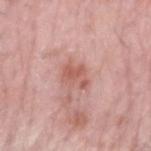Imaged during a routine full-body skin examination; the lesion was not biopsied and no histopathology is available.
The lesion-visualizer software estimated a footprint of about 5 mm², an outline eccentricity of about 0.65 (0 = round, 1 = elongated), and a symmetry-axis asymmetry near 0.35. And it measured a mean CIELAB color near L≈58 a*≈26 b*≈26 and a normalized lesion–skin contrast near 6.5. The software also gave a border-irregularity rating of about 4/10 and peripheral color asymmetry of about 0.5. The analysis additionally found a nevus-likeness score of about 20/100.
On the left forearm.
Imaged with white-light lighting.
A close-up tile cropped from a whole-body skin photograph, about 15 mm across.
A male patient, aged around 75.
Approximately 3 mm at its widest.